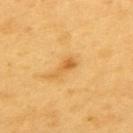follow-up=total-body-photography surveillance lesion; no biopsy
lesion size=≈3 mm
anatomic site=the upper back
acquisition=15 mm crop, total-body photography
image-analysis metrics=a shape eccentricity near 0.9
patient=male, roughly 60 years of age